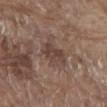follow-up — total-body-photography surveillance lesion; no biopsy
illumination — white-light illumination
body site — the front of the torso
acquisition — ~15 mm crop, total-body skin-cancer survey
image-analysis metrics — a footprint of about 11 mm² and an outline eccentricity of about 0.75 (0 = round, 1 = elongated); a lesion-to-skin contrast of about 6 (normalized; higher = more distinct)
patient — male, aged 78 to 82